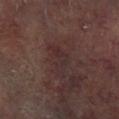{
  "biopsy_status": "not biopsied; imaged during a skin examination",
  "image": {
    "source": "total-body photography crop",
    "field_of_view_mm": 15
  },
  "patient": {
    "sex": "male",
    "age_approx": 65
  },
  "site": "left lower leg"
}